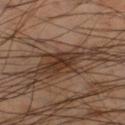Automated tile analysis of the lesion measured a normalized border contrast of about 9. The software also gave a classifier nevus-likeness of about 15/100 and lesion-presence confidence of about 55/100.
A male subject in their 50s.
Imaged with cross-polarized lighting.
Approximately 7 mm at its widest.
Located on the leg.
A 15 mm close-up extracted from a 3D total-body photography capture.